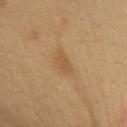Assessment: This lesion was catalogued during total-body skin photography and was not selected for biopsy. Image and clinical context: The patient is a female aged approximately 40. A 15 mm close-up extracted from a 3D total-body photography capture. The lesion is located on the chest. Longest diameter approximately 3 mm. The tile uses cross-polarized illumination.